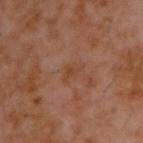The recorded lesion diameter is about 2.5 mm.
A 15 mm crop from a total-body photograph taken for skin-cancer surveillance.
Automated tile analysis of the lesion measured a footprint of about 3 mm² and a shape-asymmetry score of about 0.4 (0 = symmetric). The software also gave a lesion color around L≈40 a*≈20 b*≈30 in CIELAB and about 5 CIELAB-L* units darker than the surrounding skin. And it measured a border-irregularity index near 4/10 and a color-variation rating of about 2/10.
Imaged with cross-polarized lighting.
A male subject, in their 60s.
Located on the upper back.